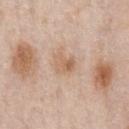Impression:
No biopsy was performed on this lesion — it was imaged during a full skin examination and was not determined to be concerning.
Image and clinical context:
This is a white-light tile. Automated image analysis of the tile measured a mean CIELAB color near L≈62 a*≈18 b*≈32, a lesion–skin lightness drop of about 8, and a lesion-to-skin contrast of about 6 (normalized; higher = more distinct). And it measured lesion-presence confidence of about 100/100. The lesion is located on the chest. A roughly 15 mm field-of-view crop from a total-body skin photograph. Measured at roughly 3 mm in maximum diameter. A male patient, aged approximately 60.